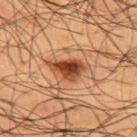workup: catalogued during a skin exam; not biopsied
acquisition: 15 mm crop, total-body photography
location: the upper back
tile lighting: cross-polarized illumination
automated lesion analysis: a footprint of about 6.5 mm², a shape eccentricity near 0.8, and a symmetry-axis asymmetry near 0.45; an average lesion color of about L≈34 a*≈22 b*≈30 (CIELAB), about 14 CIELAB-L* units darker than the surrounding skin, and a lesion-to-skin contrast of about 12.5 (normalized; higher = more distinct); internal color variation of about 5.5 on a 0–10 scale and radial color variation of about 2
subject: male, approximately 60 years of age
diameter: ≈4 mm